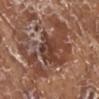Impression: Recorded during total-body skin imaging; not selected for excision or biopsy.